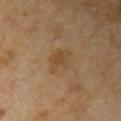Impression: The lesion was photographed on a routine skin check and not biopsied; there is no pathology result. Context: The tile uses cross-polarized illumination. The subject is a female aged around 70. An algorithmic analysis of the crop reported an area of roughly 7 mm², a shape eccentricity near 0.75, and a symmetry-axis asymmetry near 0.3. The analysis additionally found a border-irregularity index near 3/10, a within-lesion color-variation index near 4/10, and peripheral color asymmetry of about 1.5. And it measured a classifier nevus-likeness of about 5/100. A 15 mm close-up extracted from a 3D total-body photography capture. Located on the arm.